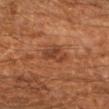Impression:
The lesion was photographed on a routine skin check and not biopsied; there is no pathology result.
Clinical summary:
From the right upper arm. A male patient, approximately 60 years of age. This is a cross-polarized tile. Cropped from a total-body skin-imaging series; the visible field is about 15 mm.